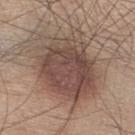Assessment: Captured during whole-body skin photography for melanoma surveillance; the lesion was not biopsied. Acquisition and patient details: A male patient, in their mid-40s. This image is a 15 mm lesion crop taken from a total-body photograph. Longest diameter approximately 9 mm. From the right lower leg. Imaged with white-light lighting. An algorithmic analysis of the crop reported a footprint of about 45 mm², an eccentricity of roughly 0.6, and a symmetry-axis asymmetry near 0.2. The software also gave a border-irregularity rating of about 3/10, internal color variation of about 6 on a 0–10 scale, and peripheral color asymmetry of about 2. The software also gave an automated nevus-likeness rating near 40 out of 100 and a lesion-detection confidence of about 100/100.